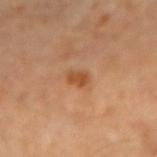Q: Was this lesion biopsied?
A: imaged on a skin check; not biopsied